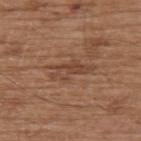Findings:
- image source: 15 mm crop, total-body photography
- subject: male, aged 73 to 77
- lesion size: ~5 mm (longest diameter)
- body site: the upper back